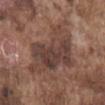This lesion was catalogued during total-body skin photography and was not selected for biopsy.
Located on the chest.
The tile uses white-light illumination.
A male patient aged 73 to 77.
A lesion tile, about 15 mm wide, cut from a 3D total-body photograph.
Longest diameter approximately 10 mm.